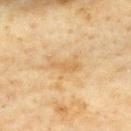Clinical impression:
This lesion was catalogued during total-body skin photography and was not selected for biopsy.
Clinical summary:
On the upper back. A 15 mm crop from a total-body photograph taken for skin-cancer surveillance. A female patient aged approximately 60. Imaged with cross-polarized lighting.